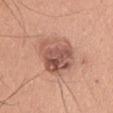workup: imaged on a skin check; not biopsied
subject: male, in their 60s
site: the chest
size: about 5 mm
tile lighting: white-light illumination
image: ~15 mm crop, total-body skin-cancer survey